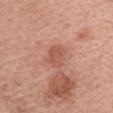This lesion was catalogued during total-body skin photography and was not selected for biopsy.
The lesion is located on the right forearm.
A 15 mm crop from a total-body photograph taken for skin-cancer surveillance.
The subject is a female in their mid-60s.
The recorded lesion diameter is about 3 mm.
Imaged with white-light lighting.